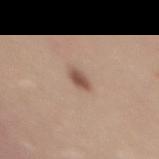<case>
<lighting>white-light</lighting>
<image>
  <source>total-body photography crop</source>
  <field_of_view_mm>15</field_of_view_mm>
</image>
<patient>
  <sex>female</sex>
  <age_approx>35</age_approx>
</patient>
<site>upper back</site>
<automated_metrics>
  <cielab_L>52</cielab_L>
  <cielab_a>19</cielab_a>
  <cielab_b>26</cielab_b>
  <vs_skin_darker_L>12.0</vs_skin_darker_L>
  <nevus_likeness_0_100>95</nevus_likeness_0_100>
</automated_metrics>
</case>